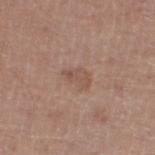Case summary:
- notes — total-body-photography surveillance lesion; no biopsy
- image source — ~15 mm crop, total-body skin-cancer survey
- image-analysis metrics — a lesion area of about 5 mm², an outline eccentricity of about 0.75 (0 = round, 1 = elongated), and two-axis asymmetry of about 0.3; a lesion color around L≈52 a*≈18 b*≈26 in CIELAB, a lesion–skin lightness drop of about 7, and a normalized border contrast of about 5; a border-irregularity index near 3/10, a color-variation rating of about 2.5/10, and a peripheral color-asymmetry measure near 1
- tile lighting — white-light
- size — ≈3 mm
- patient — male, about 60 years old
- anatomic site — the left lower leg